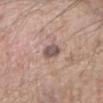{"patient": {"sex": "male", "age_approx": 60}, "lesion_size": {"long_diameter_mm_approx": 2.5}, "site": "left forearm", "image": {"source": "total-body photography crop", "field_of_view_mm": 15}}